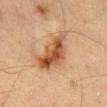Q: Was this lesion biopsied?
A: no biopsy performed (imaged during a skin exam)
Q: What lighting was used for the tile?
A: cross-polarized illumination
Q: How was this image acquired?
A: ~15 mm crop, total-body skin-cancer survey
Q: What is the anatomic site?
A: the abdomen
Q: Patient demographics?
A: male, about 60 years old
Q: Automated lesion metrics?
A: a shape eccentricity near 0.9 and a symmetry-axis asymmetry near 0.4; a border-irregularity index near 4.5/10, a within-lesion color-variation index near 7/10, and peripheral color asymmetry of about 2.5
Q: How large is the lesion?
A: about 7 mm